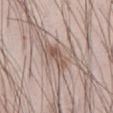Q: Is there a histopathology result?
A: total-body-photography surveillance lesion; no biopsy
Q: Where on the body is the lesion?
A: the right thigh
Q: What is the imaging modality?
A: ~15 mm tile from a whole-body skin photo
Q: What are the patient's age and sex?
A: male, in their 40s
Q: What is the lesion's diameter?
A: ~3.5 mm (longest diameter)
Q: How was the tile lit?
A: white-light